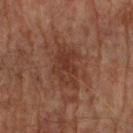Notes:
- biopsy status — no biopsy performed (imaged during a skin exam)
- imaging modality — total-body-photography crop, ~15 mm field of view
- location — the right upper arm
- patient — male, in their mid-70s